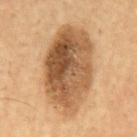notes = no biopsy performed (imaged during a skin exam)
acquisition = total-body-photography crop, ~15 mm field of view
lesion size = ~10.5 mm (longest diameter)
tile lighting = cross-polarized illumination
anatomic site = the mid back
patient = male, in their mid-50s
image-analysis metrics = a nevus-likeness score of about 75/100 and a detector confidence of about 100 out of 100 that the crop contains a lesion